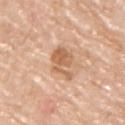{
  "biopsy_status": "not biopsied; imaged during a skin examination",
  "image": {
    "source": "total-body photography crop",
    "field_of_view_mm": 15
  },
  "patient": {
    "sex": "male",
    "age_approx": 80
  },
  "site": "upper back",
  "lighting": "white-light"
}